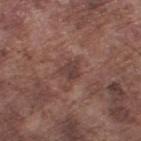| field | value |
|---|---|
| workup | no biopsy performed (imaged during a skin exam) |
| lighting | white-light |
| subject | male, in their mid-70s |
| diameter | about 2.5 mm |
| location | the right lower leg |
| image source | 15 mm crop, total-body photography |
| automated metrics | an outline eccentricity of about 0.4 (0 = round, 1 = elongated) and two-axis asymmetry of about 0.4; a border-irregularity rating of about 3.5/10, a color-variation rating of about 2.5/10, and radial color variation of about 1; a nevus-likeness score of about 0/100 and lesion-presence confidence of about 100/100 |